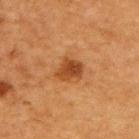follow-up = catalogued during a skin exam; not biopsied
automated lesion analysis = a mean CIELAB color near L≈40 a*≈24 b*≈36, a lesion–skin lightness drop of about 11, and a normalized lesion–skin contrast near 8.5
image source = 15 mm crop, total-body photography
tile lighting = cross-polarized
anatomic site = the upper back
lesion diameter = ≈3 mm
subject = male, aged 63–67